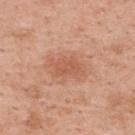Clinical impression: Imaged during a routine full-body skin examination; the lesion was not biopsied and no histopathology is available. Context: Measured at roughly 3.5 mm in maximum diameter. The tile uses white-light illumination. A female subject, in their 50s. The lesion is located on the upper back. This image is a 15 mm lesion crop taken from a total-body photograph.